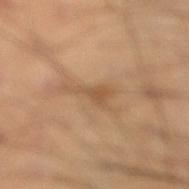<tbp_lesion>
  <biopsy_status>not biopsied; imaged during a skin examination</biopsy_status>
  <site>right lower leg</site>
  <patient>
    <sex>male</sex>
    <age_approx>40</age_approx>
  </patient>
  <image>
    <source>total-body photography crop</source>
    <field_of_view_mm>15</field_of_view_mm>
  </image>
  <lesion_size>
    <long_diameter_mm_approx>4.0</long_diameter_mm_approx>
  </lesion_size>
  <automated_metrics>
    <area_mm2_approx>4.5</area_mm2_approx>
    <eccentricity>0.85</eccentricity>
    <color_variation_0_10>2.0</color_variation_0_10>
    <peripheral_color_asymmetry>0.5</peripheral_color_asymmetry>
  </automated_metrics>
</tbp_lesion>